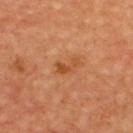notes: catalogued during a skin exam; not biopsied | image: ~15 mm tile from a whole-body skin photo | site: the upper back | patient: male, in their mid- to late 60s.